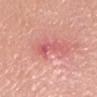  biopsy_status: not biopsied; imaged during a skin examination
  lesion_size:
    long_diameter_mm_approx: 4.0
  lighting: white-light
  image:
    source: total-body photography crop
    field_of_view_mm: 15
  patient:
    sex: female
    age_approx: 55
  site: head or neck
  automated_metrics:
    shape_asymmetry: 0.4
    cielab_L: 59
    cielab_a: 34
    cielab_b: 25
    vs_skin_darker_L: 9.0
    vs_skin_contrast_norm: 6.0
    border_irregularity_0_10: 5.0
    color_variation_0_10: 2.5
    peripheral_color_asymmetry: 0.0